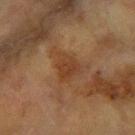Captured under cross-polarized illumination.
The subject is a female aged 58–62.
Located on the right forearm.
The lesion-visualizer software estimated a lesion area of about 6.5 mm², a shape eccentricity near 0.5, and two-axis asymmetry of about 0.3. And it measured an average lesion color of about L≈36 a*≈20 b*≈31 (CIELAB), roughly 7 lightness units darker than nearby skin, and a lesion-to-skin contrast of about 6.5 (normalized; higher = more distinct). The software also gave border irregularity of about 3 on a 0–10 scale, internal color variation of about 2.5 on a 0–10 scale, and a peripheral color-asymmetry measure near 1. And it measured a nevus-likeness score of about 25/100 and lesion-presence confidence of about 100/100.
A 15 mm close-up tile from a total-body photography series done for melanoma screening.